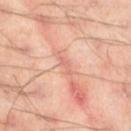notes: no biopsy performed (imaged during a skin exam)
image source: 15 mm crop, total-body photography
TBP lesion metrics: an area of roughly 2 mm², an outline eccentricity of about 0.9 (0 = round, 1 = elongated), and a symmetry-axis asymmetry near 0.4; a classifier nevus-likeness of about 0/100 and lesion-presence confidence of about 60/100
body site: the leg
diameter: about 2.5 mm
subject: male, approximately 45 years of age
tile lighting: cross-polarized illumination